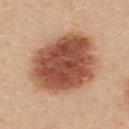Case summary:
- workup: imaged on a skin check; not biopsied
- acquisition: ~15 mm tile from a whole-body skin photo
- location: the upper back
- patient: female, aged approximately 25
- image-analysis metrics: an outline eccentricity of about 0.6 (0 = round, 1 = elongated); a lesion color around L≈52 a*≈25 b*≈32 in CIELAB, about 18 CIELAB-L* units darker than the surrounding skin, and a normalized border contrast of about 12; a classifier nevus-likeness of about 100/100
- tile lighting: white-light illumination
- lesion diameter: about 8.5 mm